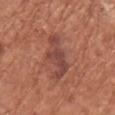Captured during whole-body skin photography for melanoma surveillance; the lesion was not biopsied.
The lesion-visualizer software estimated a footprint of about 11 mm², an eccentricity of roughly 0.9, and a shape-asymmetry score of about 0.45 (0 = symmetric). The software also gave a border-irregularity index near 6/10, a within-lesion color-variation index near 3.5/10, and radial color variation of about 1. And it measured an automated nevus-likeness rating near 45 out of 100.
On the upper back.
Longest diameter approximately 5.5 mm.
A female patient about 75 years old.
A close-up tile cropped from a whole-body skin photograph, about 15 mm across.